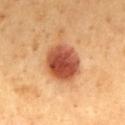| key | value |
|---|---|
| follow-up | imaged on a skin check; not biopsied |